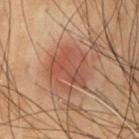A roughly 15 mm field-of-view crop from a total-body skin photograph. A male patient, approximately 55 years of age. On the chest. Captured under cross-polarized illumination.